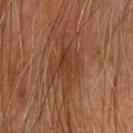Clinical impression:
This lesion was catalogued during total-body skin photography and was not selected for biopsy.
Context:
A 15 mm close-up extracted from a 3D total-body photography capture. The lesion's longest dimension is about 5 mm. The lesion is on the left upper arm. The patient is a male about 60 years old.